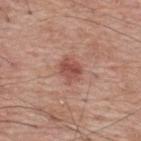Q: Was a biopsy performed?
A: no biopsy performed (imaged during a skin exam)
Q: Who is the patient?
A: male, about 70 years old
Q: Automated lesion metrics?
A: a lesion color around L≈51 a*≈25 b*≈27 in CIELAB, a lesion–skin lightness drop of about 11, and a lesion-to-skin contrast of about 7.5 (normalized; higher = more distinct); a classifier nevus-likeness of about 45/100 and lesion-presence confidence of about 100/100
Q: How was this image acquired?
A: ~15 mm crop, total-body skin-cancer survey
Q: Lesion location?
A: the upper back
Q: Lesion size?
A: ≈3 mm
Q: What lighting was used for the tile?
A: white-light illumination